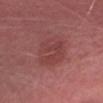<tbp_lesion>
<site>head or neck</site>
<image>
  <source>total-body photography crop</source>
  <field_of_view_mm>15</field_of_view_mm>
</image>
<lesion_size>
  <long_diameter_mm_approx>4.5</long_diameter_mm_approx>
</lesion_size>
<lighting>white-light</lighting>
<patient>
  <sex>female</sex>
  <age_approx>25</age_approx>
</patient>
</tbp_lesion>